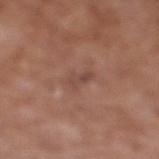Case summary:
- follow-up: imaged on a skin check; not biopsied
- patient: male, about 75 years old
- illumination: white-light illumination
- anatomic site: the right lower leg
- imaging modality: total-body-photography crop, ~15 mm field of view
- lesion diameter: ~3.5 mm (longest diameter)
- TBP lesion metrics: border irregularity of about 4.5 on a 0–10 scale and a peripheral color-asymmetry measure near 0; an automated nevus-likeness rating near 0 out of 100 and lesion-presence confidence of about 85/100